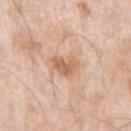follow-up: imaged on a skin check; not biopsied
image-analysis metrics: a lesion area of about 6 mm², a shape eccentricity near 0.6, and a symmetry-axis asymmetry near 0.35; a mean CIELAB color near L≈63 a*≈20 b*≈33 and a lesion–skin lightness drop of about 10; a nevus-likeness score of about 75/100
image: total-body-photography crop, ~15 mm field of view
subject: male, aged 53 to 57
anatomic site: the left upper arm
lesion diameter: ≈3 mm